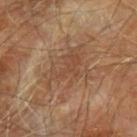* workup: no biopsy performed (imaged during a skin exam)
* automated lesion analysis: a lesion color around L≈46 a*≈19 b*≈30 in CIELAB and roughly 6 lightness units darker than nearby skin
* subject: male, in their 70s
* site: the left forearm
* illumination: cross-polarized illumination
* imaging modality: total-body-photography crop, ~15 mm field of view